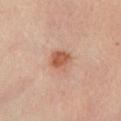Assessment:
The lesion was photographed on a routine skin check and not biopsied; there is no pathology result.
Acquisition and patient details:
From the left lower leg. The recorded lesion diameter is about 2.5 mm. A male subject, approximately 45 years of age. Automated tile analysis of the lesion measured a border-irregularity index near 1.5/10 and a color-variation rating of about 3.5/10. The analysis additionally found an automated nevus-likeness rating near 95 out of 100 and a detector confidence of about 100 out of 100 that the crop contains a lesion. A region of skin cropped from a whole-body photographic capture, roughly 15 mm wide. This is a cross-polarized tile.